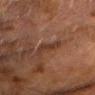Captured during whole-body skin photography for melanoma surveillance; the lesion was not biopsied.
Approximately 3.5 mm at its widest.
A 15 mm close-up extracted from a 3D total-body photography capture.
The patient is a male aged around 75.
The total-body-photography lesion software estimated an area of roughly 4.5 mm² and two-axis asymmetry of about 0.55. And it measured border irregularity of about 6 on a 0–10 scale and a color-variation rating of about 0.5/10. And it measured an automated nevus-likeness rating near 0 out of 100 and a detector confidence of about 70 out of 100 that the crop contains a lesion.
On the chest.